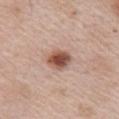Captured during whole-body skin photography for melanoma surveillance; the lesion was not biopsied.
The tile uses white-light illumination.
The lesion is on the front of the torso.
Automated image analysis of the tile measured a within-lesion color-variation index near 5/10. The analysis additionally found a nevus-likeness score of about 100/100.
The patient is a male aged around 65.
About 3 mm across.
A lesion tile, about 15 mm wide, cut from a 3D total-body photograph.